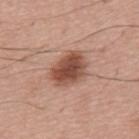Case summary:
• follow-up: total-body-photography surveillance lesion; no biopsy
• image-analysis metrics: a footprint of about 12 mm², an outline eccentricity of about 0.7 (0 = round, 1 = elongated), and two-axis asymmetry of about 0.2; a lesion color around L≈49 a*≈23 b*≈28 in CIELAB, roughly 15 lightness units darker than nearby skin, and a normalized border contrast of about 10; border irregularity of about 2 on a 0–10 scale, internal color variation of about 4.5 on a 0–10 scale, and a peripheral color-asymmetry measure near 1
• image: total-body-photography crop, ~15 mm field of view
• lesion diameter: about 4.5 mm
• patient: male, aged 53–57
• tile lighting: white-light